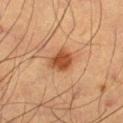<lesion>
  <biopsy_status>not biopsied; imaged during a skin examination</biopsy_status>
  <image>
    <source>total-body photography crop</source>
    <field_of_view_mm>15</field_of_view_mm>
  </image>
  <patient>
    <sex>male</sex>
    <age_approx>65</age_approx>
  </patient>
  <automated_metrics>
    <area_mm2_approx>6.0</area_mm2_approx>
    <eccentricity>0.55</eccentricity>
    <shape_asymmetry>0.25</shape_asymmetry>
  </automated_metrics>
  <lighting>cross-polarized</lighting>
  <site>right thigh</site>
  <lesion_size>
    <long_diameter_mm_approx>3.0</long_diameter_mm_approx>
  </lesion_size>
</lesion>